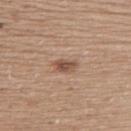Captured during whole-body skin photography for melanoma surveillance; the lesion was not biopsied. About 3 mm across. The lesion is located on the upper back. A 15 mm close-up tile from a total-body photography series done for melanoma screening. The lesion-visualizer software estimated an average lesion color of about L≈51 a*≈19 b*≈28 (CIELAB), a lesion–skin lightness drop of about 11, and a normalized lesion–skin contrast near 8. The analysis additionally found border irregularity of about 2 on a 0–10 scale and a peripheral color-asymmetry measure near 1.5. The tile uses white-light illumination. The patient is a female approximately 60 years of age.